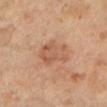| feature | finding |
|---|---|
| follow-up | imaged on a skin check; not biopsied |
| acquisition | total-body-photography crop, ~15 mm field of view |
| lighting | cross-polarized |
| lesion size | ≈4.5 mm |
| site | the left lower leg |
| patient | female, approximately 70 years of age |
| automated lesion analysis | a lesion area of about 10 mm² and a symmetry-axis asymmetry near 0.3; an automated nevus-likeness rating near 20 out of 100 and a detector confidence of about 100 out of 100 that the crop contains a lesion |